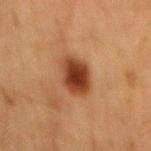Impression: Recorded during total-body skin imaging; not selected for excision or biopsy. Background: From the back. A male subject approximately 65 years of age. A close-up tile cropped from a whole-body skin photograph, about 15 mm across.